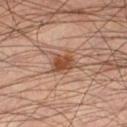{"biopsy_status": "not biopsied; imaged during a skin examination", "image": {"source": "total-body photography crop", "field_of_view_mm": 15}, "patient": {"sex": "male", "age_approx": 45}, "site": "left lower leg", "lesion_size": {"long_diameter_mm_approx": 3.0}, "lighting": "cross-polarized"}